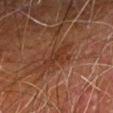Part of a total-body skin-imaging series; this lesion was reviewed on a skin check and was not flagged for biopsy.
The lesion is on the right forearm.
Captured under cross-polarized illumination.
A male subject, approximately 80 years of age.
This image is a 15 mm lesion crop taken from a total-body photograph.